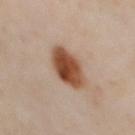From the chest.
A male subject about 65 years old.
Cropped from a total-body skin-imaging series; the visible field is about 15 mm.
The biopsy diagnosis was a compound melanocytic nevus.